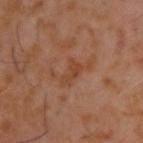{"biopsy_status": "not biopsied; imaged during a skin examination", "lesion_size": {"long_diameter_mm_approx": 5.0}, "image": {"source": "total-body photography crop", "field_of_view_mm": 15}, "lighting": "cross-polarized", "automated_metrics": {"border_irregularity_0_10": 8.5, "peripheral_color_asymmetry": 1.0}, "site": "upper back", "patient": {"sex": "male", "age_approx": 60}}